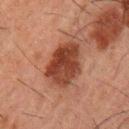<tbp_lesion>
  <biopsy_status>not biopsied; imaged during a skin examination</biopsy_status>
  <site>left upper arm</site>
  <lesion_size>
    <long_diameter_mm_approx>5.0</long_diameter_mm_approx>
  </lesion_size>
  <patient>
    <sex>male</sex>
    <age_approx>50</age_approx>
  </patient>
  <image>
    <source>total-body photography crop</source>
    <field_of_view_mm>15</field_of_view_mm>
  </image>
</tbp_lesion>